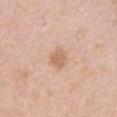Captured during whole-body skin photography for melanoma surveillance; the lesion was not biopsied.
Captured under white-light illumination.
From the chest.
Longest diameter approximately 2.5 mm.
A region of skin cropped from a whole-body photographic capture, roughly 15 mm wide.
A female subject, aged around 60.